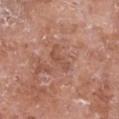workup: catalogued during a skin exam; not biopsied
lesion diameter: ~3.5 mm (longest diameter)
location: the front of the torso
image: 15 mm crop, total-body photography
patient: female, aged 73 to 77
illumination: white-light illumination